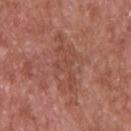No biopsy was performed on this lesion — it was imaged during a full skin examination and was not determined to be concerning. Cropped from a total-body skin-imaging series; the visible field is about 15 mm. Measured at roughly 6.5 mm in maximum diameter. Captured under white-light illumination. The lesion is on the left upper arm. Automated tile analysis of the lesion measured a lesion area of about 13 mm², a shape eccentricity near 0.9, and a shape-asymmetry score of about 0.4 (0 = symmetric). The software also gave a mean CIELAB color near L≈47 a*≈24 b*≈28, a lesion–skin lightness drop of about 6, and a normalized lesion–skin contrast near 4.5. The software also gave a border-irregularity index near 7/10, a color-variation rating of about 3/10, and peripheral color asymmetry of about 1. The analysis additionally found a classifier nevus-likeness of about 0/100 and a detector confidence of about 85 out of 100 that the crop contains a lesion. A male subject, in their mid- to late 60s.